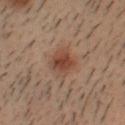The lesion is on the head or neck.
Longest diameter approximately 4 mm.
The total-body-photography lesion software estimated an area of roughly 9 mm² and a shape eccentricity near 0.6. It also reported a lesion color around L≈36 a*≈17 b*≈24 in CIELAB, a lesion–skin lightness drop of about 7, and a lesion-to-skin contrast of about 7 (normalized; higher = more distinct). The analysis additionally found a border-irregularity rating of about 2/10.
A 15 mm crop from a total-body photograph taken for skin-cancer surveillance.
Imaged with cross-polarized lighting.
A male subject, in their 30s.